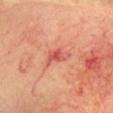Case summary:
- biopsy status: catalogued during a skin exam; not biopsied
- lesion size: ~3.5 mm (longest diameter)
- image: ~15 mm tile from a whole-body skin photo
- subject: female, in their mid-70s
- illumination: cross-polarized illumination
- anatomic site: the head or neck